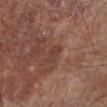No biopsy was performed on this lesion — it was imaged during a full skin examination and was not determined to be concerning.
A male subject, roughly 70 years of age.
Automated image analysis of the tile measured a lesion area of about 2.5 mm², an outline eccentricity of about 0.85 (0 = round, 1 = elongated), and two-axis asymmetry of about 0.5. It also reported a mean CIELAB color near L≈39 a*≈21 b*≈23 and a lesion-to-skin contrast of about 5.5 (normalized; higher = more distinct). The software also gave internal color variation of about 0 on a 0–10 scale and a peripheral color-asymmetry measure near 0. And it measured an automated nevus-likeness rating near 0 out of 100 and a detector confidence of about 100 out of 100 that the crop contains a lesion.
The lesion is located on the left lower leg.
Approximately 2.5 mm at its widest.
A 15 mm crop from a total-body photograph taken for skin-cancer surveillance.
Captured under white-light illumination.